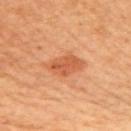| field | value |
|---|---|
| workup | total-body-photography surveillance lesion; no biopsy |
| tile lighting | cross-polarized illumination |
| subject | female, approximately 40 years of age |
| body site | the right upper arm |
| image | total-body-photography crop, ~15 mm field of view |